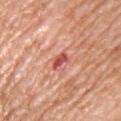The lesion was photographed on a routine skin check and not biopsied; there is no pathology result. This image is a 15 mm lesion crop taken from a total-body photograph. Automated image analysis of the tile measured about 14 CIELAB-L* units darker than the surrounding skin. And it measured a border-irregularity index near 3.5/10 and a peripheral color-asymmetry measure near 0. The software also gave a nevus-likeness score of about 0/100 and a lesion-detection confidence of about 100/100. Located on the chest. The subject is a male aged 63–67. Approximately 2.5 mm at its widest. Captured under white-light illumination.